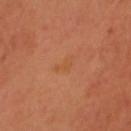{
  "biopsy_status": "not biopsied; imaged during a skin examination",
  "patient": {
    "sex": "male",
    "age_approx": 55
  },
  "image": {
    "source": "total-body photography crop",
    "field_of_view_mm": 15
  },
  "site": "head or neck"
}